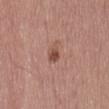{
  "biopsy_status": "not biopsied; imaged during a skin examination",
  "patient": {
    "sex": "male",
    "age_approx": 75
  },
  "lesion_size": {
    "long_diameter_mm_approx": 2.5
  },
  "lighting": "white-light",
  "image": {
    "source": "total-body photography crop",
    "field_of_view_mm": 15
  },
  "site": "leg",
  "automated_metrics": {
    "area_mm2_approx": 3.5,
    "eccentricity": 0.85,
    "shape_asymmetry": 0.2,
    "cielab_L": 50,
    "cielab_a": 22,
    "cielab_b": 27,
    "vs_skin_darker_L": 10.0,
    "color_variation_0_10": 4.0,
    "peripheral_color_asymmetry": 1.5
  }
}